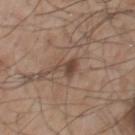{
  "biopsy_status": "not biopsied; imaged during a skin examination",
  "lighting": "white-light",
  "image": {
    "source": "total-body photography crop",
    "field_of_view_mm": 15
  },
  "lesion_size": {
    "long_diameter_mm_approx": 3.5
  },
  "site": "chest",
  "patient": {
    "sex": "male",
    "age_approx": 60
  }
}